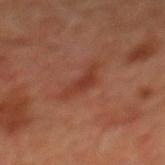This lesion was catalogued during total-body skin photography and was not selected for biopsy. A 15 mm close-up tile from a total-body photography series done for melanoma screening. The patient is a male roughly 60 years of age. From the chest.